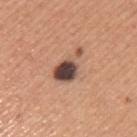This lesion was catalogued during total-body skin photography and was not selected for biopsy. Located on the left upper arm. Approximately 5 mm at its widest. An algorithmic analysis of the crop reported a lesion color around L≈47 a*≈19 b*≈24 in CIELAB. The software also gave a nevus-likeness score of about 25/100 and a lesion-detection confidence of about 100/100. Imaged with white-light lighting. The patient is a male roughly 45 years of age. A 15 mm close-up extracted from a 3D total-body photography capture.